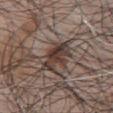Assessment:
The lesion was tiled from a total-body skin photograph and was not biopsied.
Clinical summary:
A male patient aged approximately 50. The tile uses white-light illumination. Approximately 5 mm at its widest. The lesion is on the chest. A lesion tile, about 15 mm wide, cut from a 3D total-body photograph.